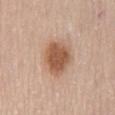<case>
  <image>
    <source>total-body photography crop</source>
    <field_of_view_mm>15</field_of_view_mm>
  </image>
  <patient>
    <sex>female</sex>
    <age_approx>70</age_approx>
  </patient>
  <lesion_size>
    <long_diameter_mm_approx>5.0</long_diameter_mm_approx>
  </lesion_size>
  <lighting>white-light</lighting>
  <site>mid back</site>
</case>